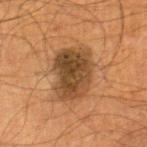Measured at roughly 6 mm in maximum diameter.
A lesion tile, about 15 mm wide, cut from a 3D total-body photograph.
Imaged with cross-polarized lighting.
A male subject aged 58–62.
The total-body-photography lesion software estimated a shape eccentricity near 0.75 and two-axis asymmetry of about 0.15. The software also gave a border-irregularity rating of about 2/10, internal color variation of about 5.5 on a 0–10 scale, and a peripheral color-asymmetry measure near 2.
The lesion is located on the right thigh.